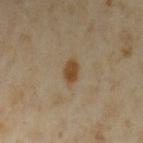<case>
  <biopsy_status>not biopsied; imaged during a skin examination</biopsy_status>
  <patient>
    <sex>female</sex>
    <age_approx>35</age_approx>
  </patient>
  <lesion_size>
    <long_diameter_mm_approx>3.0</long_diameter_mm_approx>
  </lesion_size>
  <automated_metrics>
    <area_mm2_approx>4.0</area_mm2_approx>
    <shape_asymmetry>0.2</shape_asymmetry>
    <cielab_L>43</cielab_L>
    <cielab_a>15</cielab_a>
    <cielab_b>33</cielab_b>
    <vs_skin_darker_L>10.0</vs_skin_darker_L>
    <vs_skin_contrast_norm>10.0</vs_skin_contrast_norm>
    <border_irregularity_0_10>2.0</border_irregularity_0_10>
    <color_variation_0_10>2.0</color_variation_0_10>
    <peripheral_color_asymmetry>0.5</peripheral_color_asymmetry>
  </automated_metrics>
  <site>left upper arm</site>
  <image>
    <source>total-body photography crop</source>
    <field_of_view_mm>15</field_of_view_mm>
  </image>
  <lighting>cross-polarized</lighting>
</case>